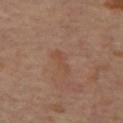The lesion was tiled from a total-body skin photograph and was not biopsied. This image is a 15 mm lesion crop taken from a total-body photograph. The lesion-visualizer software estimated a lesion area of about 4 mm², an eccentricity of roughly 0.9, and a symmetry-axis asymmetry near 0.45. The tile uses cross-polarized illumination. A female subject aged 43 to 47. On the back.